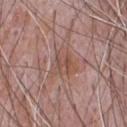| key | value |
|---|---|
| biopsy status | no biopsy performed (imaged during a skin exam) |
| site | the chest |
| patient | male, in their 70s |
| automated lesion analysis | an automated nevus-likeness rating near 0 out of 100 and lesion-presence confidence of about 75/100 |
| image source | total-body-photography crop, ~15 mm field of view |